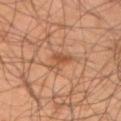The lesion was tiled from a total-body skin photograph and was not biopsied.
Automated image analysis of the tile measured an automated nevus-likeness rating near 45 out of 100 and a detector confidence of about 100 out of 100 that the crop contains a lesion.
A roughly 15 mm field-of-view crop from a total-body skin photograph.
The tile uses cross-polarized illumination.
On the left upper arm.
The recorded lesion diameter is about 3.5 mm.
A male subject aged around 45.